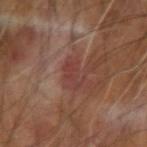{"biopsy_status": "not biopsied; imaged during a skin examination", "site": "right arm", "image": {"source": "total-body photography crop", "field_of_view_mm": 15}, "patient": {"sex": "male", "age_approx": 60}}